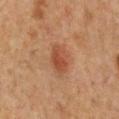biopsy status: catalogued during a skin exam; not biopsied
location: the front of the torso
TBP lesion metrics: an average lesion color of about L≈38 a*≈22 b*≈28 (CIELAB), roughly 8 lightness units darker than nearby skin, and a normalized lesion–skin contrast near 7; border irregularity of about 2 on a 0–10 scale, a color-variation rating of about 3/10, and radial color variation of about 1
tile lighting: cross-polarized
imaging modality: 15 mm crop, total-body photography
subject: male, in their 50s
diameter: ≈4 mm